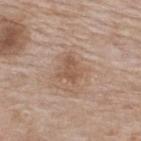{
  "biopsy_status": "not biopsied; imaged during a skin examination",
  "patient": {
    "sex": "female",
    "age_approx": 75
  },
  "site": "upper back",
  "image": {
    "source": "total-body photography crop",
    "field_of_view_mm": 15
  }
}